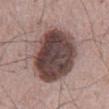Q: Was this lesion biopsied?
A: no biopsy performed (imaged during a skin exam)
Q: What lighting was used for the tile?
A: white-light
Q: Patient demographics?
A: male, approximately 65 years of age
Q: What did automated image analysis measure?
A: an outline eccentricity of about 0.8 (0 = round, 1 = elongated) and a symmetry-axis asymmetry near 0.1; a lesion–skin lightness drop of about 19 and a normalized border contrast of about 13.5; a classifier nevus-likeness of about 5/100 and a lesion-detection confidence of about 100/100
Q: Lesion size?
A: ~9 mm (longest diameter)
Q: Lesion location?
A: the mid back
Q: What is the imaging modality?
A: ~15 mm tile from a whole-body skin photo